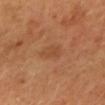  biopsy_status: not biopsied; imaged during a skin examination
  automated_metrics:
    eccentricity: 0.8
    shape_asymmetry: 0.25
    cielab_L: 49
    cielab_a: 24
    cielab_b: 36
    vs_skin_darker_L: 6.0
    vs_skin_contrast_norm: 5.0
  site: mid back
  image:
    source: total-body photography crop
    field_of_view_mm: 15
  lighting: cross-polarized
  patient:
    sex: female
    age_approx: 60